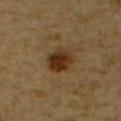Imaged during a routine full-body skin examination; the lesion was not biopsied and no histopathology is available. Located on the right upper arm. A 15 mm close-up extracted from a 3D total-body photography capture. Imaged with cross-polarized lighting. A male subject, roughly 85 years of age or older. Approximately 3.5 mm at its widest.